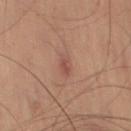Imaged during a routine full-body skin examination; the lesion was not biopsied and no histopathology is available. A male patient roughly 50 years of age. From the left thigh. A 15 mm close-up tile from a total-body photography series done for melanoma screening.